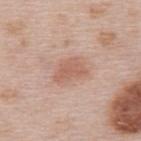The lesion was tiled from a total-body skin photograph and was not biopsied. Captured under white-light illumination. Longest diameter approximately 4 mm. On the upper back. A female patient, in their mid-60s. An algorithmic analysis of the crop reported a lesion area of about 7.5 mm², an eccentricity of roughly 0.75, and a shape-asymmetry score of about 0.35 (0 = symmetric). The software also gave a mean CIELAB color near L≈60 a*≈22 b*≈28 and roughly 9 lightness units darker than nearby skin. It also reported a border-irregularity index near 3.5/10, internal color variation of about 2 on a 0–10 scale, and a peripheral color-asymmetry measure near 0.5. A 15 mm close-up extracted from a 3D total-body photography capture.